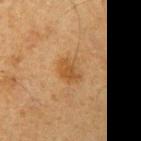The lesion was photographed on a routine skin check and not biopsied; there is no pathology result.
A male patient, aged 63–67.
From the left upper arm.
Cropped from a total-body skin-imaging series; the visible field is about 15 mm.
Automated image analysis of the tile measured a lesion color around L≈43 a*≈17 b*≈34 in CIELAB.
About 3 mm across.
Captured under cross-polarized illumination.